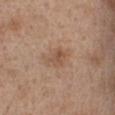Clinical impression:
The lesion was tiled from a total-body skin photograph and was not biopsied.
Image and clinical context:
An algorithmic analysis of the crop reported two-axis asymmetry of about 0.25. The software also gave an average lesion color of about L≈52 a*≈19 b*≈30 (CIELAB), a lesion–skin lightness drop of about 8, and a normalized lesion–skin contrast near 6. The analysis additionally found a classifier nevus-likeness of about 15/100. A female patient aged around 40. About 3 mm across. The lesion is on the chest. Cropped from a whole-body photographic skin survey; the tile spans about 15 mm. Imaged with white-light lighting.